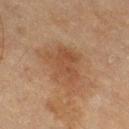Q: What is the imaging modality?
A: 15 mm crop, total-body photography
Q: How large is the lesion?
A: about 5 mm
Q: Who is the patient?
A: female, roughly 60 years of age
Q: Illumination type?
A: cross-polarized illumination
Q: Lesion location?
A: the right thigh